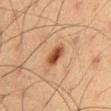biopsy status = no biopsy performed (imaged during a skin exam) | site = the mid back | tile lighting = cross-polarized illumination | size = ≈3 mm | patient = male, aged 58 to 62 | image source = ~15 mm crop, total-body skin-cancer survey.